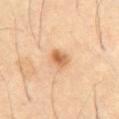Impression: Recorded during total-body skin imaging; not selected for excision or biopsy. Image and clinical context: The lesion is on the abdomen. A lesion tile, about 15 mm wide, cut from a 3D total-body photograph. About 2.5 mm across. A male patient, approximately 55 years of age. The tile uses cross-polarized illumination.